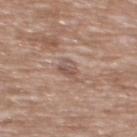Case summary:
• follow-up: catalogued during a skin exam; not biopsied
• site: the upper back
• patient: female, about 75 years old
• lesion diameter: about 2.5 mm
• image source: ~15 mm tile from a whole-body skin photo